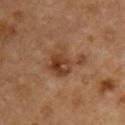Q: What did automated image analysis measure?
A: an average lesion color of about L≈40 a*≈21 b*≈32 (CIELAB), about 9 CIELAB-L* units darker than the surrounding skin, and a normalized lesion–skin contrast near 7.5
Q: Patient demographics?
A: male, in their mid- to late 50s
Q: How was the tile lit?
A: cross-polarized
Q: Lesion size?
A: ≈5 mm
Q: What is the anatomic site?
A: the chest
Q: How was this image acquired?
A: ~15 mm tile from a whole-body skin photo